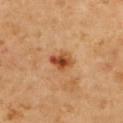Q: Was this lesion biopsied?
A: total-body-photography surveillance lesion; no biopsy
Q: What is the imaging modality?
A: ~15 mm tile from a whole-body skin photo
Q: What is the anatomic site?
A: the upper back
Q: How was the tile lit?
A: cross-polarized
Q: What did automated image analysis measure?
A: an average lesion color of about L≈51 a*≈28 b*≈40 (CIELAB) and a normalized lesion–skin contrast near 9.5; a nevus-likeness score of about 55/100 and lesion-presence confidence of about 100/100
Q: What is the lesion's diameter?
A: ≈3 mm
Q: Who is the patient?
A: female, roughly 45 years of age